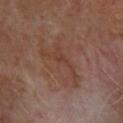Q: Was this lesion biopsied?
A: catalogued during a skin exam; not biopsied
Q: Patient demographics?
A: male, aged 63 to 67
Q: Lesion location?
A: the upper back
Q: What did automated image analysis measure?
A: a footprint of about 7.5 mm², an eccentricity of roughly 0.9, and two-axis asymmetry of about 0.75; a color-variation rating of about 1.5/10
Q: Illumination type?
A: cross-polarized illumination
Q: How was this image acquired?
A: ~15 mm tile from a whole-body skin photo